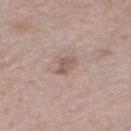  biopsy_status: not biopsied; imaged during a skin examination